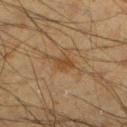{
  "biopsy_status": "not biopsied; imaged during a skin examination",
  "image": {
    "source": "total-body photography crop",
    "field_of_view_mm": 15
  },
  "site": "left lower leg",
  "lesion_size": {
    "long_diameter_mm_approx": 3.0
  },
  "patient": {
    "sex": "male",
    "age_approx": 35
  },
  "lighting": "cross-polarized"
}